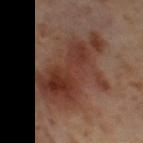subject: female, approximately 55 years of age
diameter: ~9 mm (longest diameter)
image source: 15 mm crop, total-body photography
TBP lesion metrics: a border-irregularity index near 4.5/10 and peripheral color asymmetry of about 2.5; a classifier nevus-likeness of about 10/100 and lesion-presence confidence of about 100/100
anatomic site: the right thigh